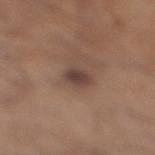Clinical impression:
No biopsy was performed on this lesion — it was imaged during a full skin examination and was not determined to be concerning.
Background:
A male subject, approximately 60 years of age. The tile uses white-light illumination. Measured at roughly 2.5 mm in maximum diameter. A 15 mm close-up extracted from a 3D total-body photography capture. Located on the right lower leg. An algorithmic analysis of the crop reported a footprint of about 4 mm², an eccentricity of roughly 0.75, and a shape-asymmetry score of about 0.2 (0 = symmetric). The analysis additionally found an average lesion color of about L≈41 a*≈16 b*≈22 (CIELAB) and about 10 CIELAB-L* units darker than the surrounding skin. The analysis additionally found a border-irregularity index near 1.5/10 and a peripheral color-asymmetry measure near 1. It also reported an automated nevus-likeness rating near 65 out of 100 and a lesion-detection confidence of about 100/100.